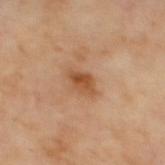Q: Was this lesion biopsied?
A: total-body-photography surveillance lesion; no biopsy
Q: Lesion size?
A: about 3 mm
Q: What did automated image analysis measure?
A: an area of roughly 4.5 mm², an eccentricity of roughly 0.8, and a symmetry-axis asymmetry near 0.2; a mean CIELAB color near L≈52 a*≈23 b*≈37, a lesion–skin lightness drop of about 11, and a lesion-to-skin contrast of about 8 (normalized; higher = more distinct)
Q: Illumination type?
A: cross-polarized illumination
Q: What is the imaging modality?
A: ~15 mm tile from a whole-body skin photo
Q: Where on the body is the lesion?
A: the mid back